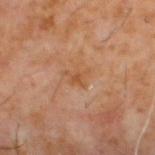The lesion was tiled from a total-body skin photograph and was not biopsied.
A roughly 15 mm field-of-view crop from a total-body skin photograph.
The patient is a male approximately 60 years of age.
Located on the upper back.
Measured at roughly 3 mm in maximum diameter.
An algorithmic analysis of the crop reported a border-irregularity index near 6.5/10, internal color variation of about 1 on a 0–10 scale, and radial color variation of about 0.5. It also reported an automated nevus-likeness rating near 0 out of 100 and a lesion-detection confidence of about 100/100.